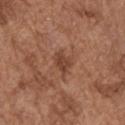Assessment:
No biopsy was performed on this lesion — it was imaged during a full skin examination and was not determined to be concerning.
Image and clinical context:
Approximately 3.5 mm at its widest. A male subject aged approximately 75. A 15 mm crop from a total-body photograph taken for skin-cancer surveillance. The lesion is on the arm. This is a white-light tile. The lesion-visualizer software estimated a lesion area of about 5 mm², an eccentricity of roughly 0.7, and two-axis asymmetry of about 0.35. It also reported a lesion color around L≈43 a*≈22 b*≈29 in CIELAB, about 9 CIELAB-L* units darker than the surrounding skin, and a normalized lesion–skin contrast near 7.